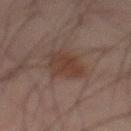<record>
  <biopsy_status>not biopsied; imaged during a skin examination</biopsy_status>
  <automated_metrics>
    <area_mm2_approx>10.0</area_mm2_approx>
    <shape_asymmetry>0.25</shape_asymmetry>
    <vs_skin_darker_L>6.0</vs_skin_darker_L>
    <vs_skin_contrast_norm>7.0</vs_skin_contrast_norm>
    <border_irregularity_0_10>2.5</border_irregularity_0_10>
    <color_variation_0_10>3.0</color_variation_0_10>
    <peripheral_color_asymmetry>1.0</peripheral_color_asymmetry>
    <nevus_likeness_0_100>75</nevus_likeness_0_100>
    <lesion_detection_confidence_0_100>100</lesion_detection_confidence_0_100>
  </automated_metrics>
  <lesion_size>
    <long_diameter_mm_approx>4.0</long_diameter_mm_approx>
  </lesion_size>
  <site>mid back</site>
  <patient>
    <sex>male</sex>
    <age_approx>65</age_approx>
  </patient>
  <image>
    <source>total-body photography crop</source>
    <field_of_view_mm>15</field_of_view_mm>
  </image>
</record>